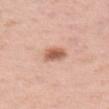{
  "biopsy_status": "not biopsied; imaged during a skin examination",
  "image": {
    "source": "total-body photography crop",
    "field_of_view_mm": 15
  },
  "patient": {
    "sex": "female",
    "age_approx": 50
  },
  "lesion_size": {
    "long_diameter_mm_approx": 3.5
  },
  "site": "upper back",
  "lighting": "white-light",
  "automated_metrics": {
    "cielab_L": 60,
    "cielab_a": 23,
    "cielab_b": 31,
    "vs_skin_darker_L": 14.0,
    "vs_skin_contrast_norm": 9.0,
    "border_irregularity_0_10": 2.0,
    "color_variation_0_10": 3.0,
    "peripheral_color_asymmetry": 1.0,
    "nevus_likeness_0_100": 95,
    "lesion_detection_confidence_0_100": 100
  }
}